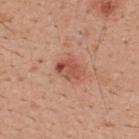<lesion>
<biopsy_status>not biopsied; imaged during a skin examination</biopsy_status>
<patient>
  <sex>male</sex>
  <age_approx>30</age_approx>
</patient>
<image>
  <source>total-body photography crop</source>
  <field_of_view_mm>15</field_of_view_mm>
</image>
<site>upper back</site>
</lesion>